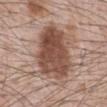<lesion>
<lighting>white-light</lighting>
<patient>
  <sex>male</sex>
  <age_approx>70</age_approx>
</patient>
<automated_metrics>
  <nevus_likeness_0_100>65</nevus_likeness_0_100>
  <lesion_detection_confidence_0_100>100</lesion_detection_confidence_0_100>
</automated_metrics>
<image>
  <source>total-body photography crop</source>
  <field_of_view_mm>15</field_of_view_mm>
</image>
<site>abdomen</site>
<lesion_size>
  <long_diameter_mm_approx>7.0</long_diameter_mm_approx>
</lesion_size>
</lesion>